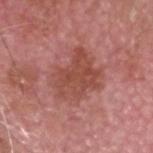Recorded during total-body skin imaging; not selected for excision or biopsy.
From the head or neck.
A male subject aged around 75.
A 15 mm close-up tile from a total-body photography series done for melanoma screening.